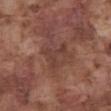{"biopsy_status": "not biopsied; imaged during a skin examination", "patient": {"sex": "male", "age_approx": 75}, "site": "abdomen", "image": {"source": "total-body photography crop", "field_of_view_mm": 15}, "lighting": "white-light", "lesion_size": {"long_diameter_mm_approx": 3.5}}